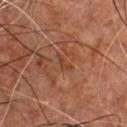  biopsy_status: not biopsied; imaged during a skin examination
  patient:
    sex: male
    age_approx: 50
  site: chest
  image:
    source: total-body photography crop
    field_of_view_mm: 15